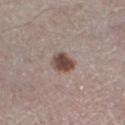illumination=white-light illumination | size=≈3 mm | subject=male, aged 63 to 67 | anatomic site=the left lower leg | image source=total-body-photography crop, ~15 mm field of view | image-analysis metrics=an eccentricity of roughly 0.6 and two-axis asymmetry of about 0.15.